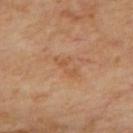Assessment: Part of a total-body skin-imaging series; this lesion was reviewed on a skin check and was not flagged for biopsy. Context: A close-up tile cropped from a whole-body skin photograph, about 15 mm across. Automated tile analysis of the lesion measured roughly 6 lightness units darker than nearby skin and a normalized border contrast of about 5. And it measured a border-irregularity rating of about 8.5/10, internal color variation of about 0 on a 0–10 scale, and radial color variation of about 0. This is a cross-polarized tile. On the upper back. A male subject in their 70s.